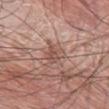Assessment:
The lesion was tiled from a total-body skin photograph and was not biopsied.
Context:
Measured at roughly 3 mm in maximum diameter. An algorithmic analysis of the crop reported a mean CIELAB color near L≈52 a*≈20 b*≈24, about 9 CIELAB-L* units darker than the surrounding skin, and a normalized border contrast of about 6. A 15 mm close-up tile from a total-body photography series done for melanoma screening. A male patient about 75 years old. On the left thigh.